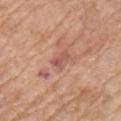workup: catalogued during a skin exam; not biopsied
image-analysis metrics: a footprint of about 3 mm² and a symmetry-axis asymmetry near 0.4; a lesion color around L≈54 a*≈25 b*≈27 in CIELAB, a lesion–skin lightness drop of about 9, and a normalized lesion–skin contrast near 6; an automated nevus-likeness rating near 0 out of 100 and lesion-presence confidence of about 100/100
imaging modality: 15 mm crop, total-body photography
body site: the chest
lesion size: about 2.5 mm
patient: female, approximately 65 years of age